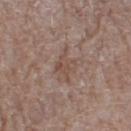biopsy_status: not biopsied; imaged during a skin examination
lighting: white-light
site: left thigh
lesion_size:
  long_diameter_mm_approx: 3.5
image:
  source: total-body photography crop
  field_of_view_mm: 15
patient:
  sex: male
  age_approx: 80